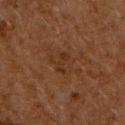Notes:
* follow-up — total-body-photography surveillance lesion; no biopsy